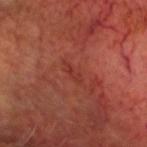Assessment:
The lesion was photographed on a routine skin check and not biopsied; there is no pathology result.
Clinical summary:
The recorded lesion diameter is about 2.5 mm. Cropped from a whole-body photographic skin survey; the tile spans about 15 mm. A male patient, in their mid-60s. Located on the head or neck. Captured under cross-polarized illumination.